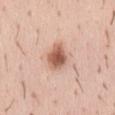Part of a total-body skin-imaging series; this lesion was reviewed on a skin check and was not flagged for biopsy. Measured at roughly 3.5 mm in maximum diameter. A 15 mm crop from a total-body photograph taken for skin-cancer surveillance. This is a white-light tile. The lesion is located on the abdomen. A female patient about 55 years old.